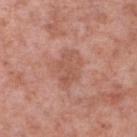follow-up — imaged on a skin check; not biopsied
image source — ~15 mm crop, total-body skin-cancer survey
automated metrics — an average lesion color of about L≈55 a*≈24 b*≈29 (CIELAB)
subject — male, about 55 years old
body site — the right lower leg
illumination — white-light